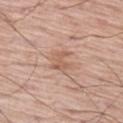• follow-up: catalogued during a skin exam; not biopsied
• anatomic site: the leg
• subject: male, aged 68–72
• tile lighting: white-light
• automated lesion analysis: a color-variation rating of about 1/10 and peripheral color asymmetry of about 0.5
• image source: total-body-photography crop, ~15 mm field of view